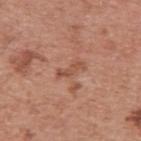Q: Was a biopsy performed?
A: catalogued during a skin exam; not biopsied
Q: What are the patient's age and sex?
A: male, aged around 55
Q: What is the imaging modality?
A: ~15 mm tile from a whole-body skin photo
Q: Lesion location?
A: the upper back